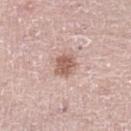Clinical impression:
Part of a total-body skin-imaging series; this lesion was reviewed on a skin check and was not flagged for biopsy.
Background:
Cropped from a whole-body photographic skin survey; the tile spans about 15 mm. From the right leg. A male patient aged 68 to 72. Automated image analysis of the tile measured an average lesion color of about L≈58 a*≈20 b*≈26 (CIELAB), about 13 CIELAB-L* units darker than the surrounding skin, and a normalized border contrast of about 8.5. The lesion's longest dimension is about 2.5 mm. Captured under white-light illumination.